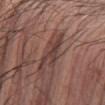Recorded during total-body skin imaging; not selected for excision or biopsy. A 15 mm close-up extracted from a 3D total-body photography capture. Approximately 4.5 mm at its widest. From the arm. Captured under white-light illumination. The patient is a male aged around 65.